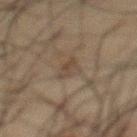A lesion tile, about 15 mm wide, cut from a 3D total-body photograph. The subject is a male aged 58 to 62. Captured under cross-polarized illumination. The lesion's longest dimension is about 2.5 mm. An algorithmic analysis of the crop reported an area of roughly 3.5 mm², an eccentricity of roughly 0.75, and two-axis asymmetry of about 0.25. The analysis additionally found a lesion–skin lightness drop of about 5 and a normalized border contrast of about 5.5. And it measured border irregularity of about 3.5 on a 0–10 scale and a peripheral color-asymmetry measure near 0.5. And it measured a classifier nevus-likeness of about 0/100 and a lesion-detection confidence of about 95/100. The lesion is on the chest.